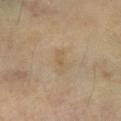{
  "biopsy_status": "not biopsied; imaged during a skin examination",
  "image": {
    "source": "total-body photography crop",
    "field_of_view_mm": 15
  },
  "patient": {
    "sex": "male",
    "age_approx": 65
  },
  "lesion_size": {
    "long_diameter_mm_approx": 3.0
  },
  "automated_metrics": {
    "border_irregularity_0_10": 4.0,
    "nevus_likeness_0_100": 0,
    "lesion_detection_confidence_0_100": 100
  },
  "lighting": "cross-polarized",
  "site": "leg"
}